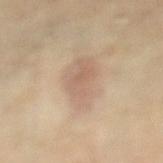<tbp_lesion>
<biopsy_status>not biopsied; imaged during a skin examination</biopsy_status>
<lesion_size>
  <long_diameter_mm_approx>5.5</long_diameter_mm_approx>
</lesion_size>
<automated_metrics>
  <lesion_detection_confidence_0_100>100</lesion_detection_confidence_0_100>
</automated_metrics>
<patient>
  <sex>female</sex>
  <age_approx>75</age_approx>
</patient>
<lighting>cross-polarized</lighting>
<site>right thigh</site>
<image>
  <source>total-body photography crop</source>
  <field_of_view_mm>15</field_of_view_mm>
</image>
</tbp_lesion>